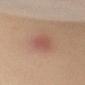Part of a total-body skin-imaging series; this lesion was reviewed on a skin check and was not flagged for biopsy. A female patient, aged around 55. Imaged with cross-polarized lighting. The lesion is on the right upper arm. This image is a 15 mm lesion crop taken from a total-body photograph. The lesion-visualizer software estimated roughly 8 lightness units darker than nearby skin. And it measured a border-irregularity rating of about 1.5/10 and peripheral color asymmetry of about 0.5. It also reported an automated nevus-likeness rating near 5 out of 100 and lesion-presence confidence of about 100/100.